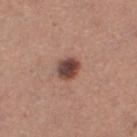| field | value |
|---|---|
| biopsy status | imaged on a skin check; not biopsied |
| TBP lesion metrics | an outline eccentricity of about 0.45 (0 = round, 1 = elongated) and two-axis asymmetry of about 0.2 |
| lighting | white-light illumination |
| subject | female, aged 28–32 |
| body site | the right thigh |
| image | 15 mm crop, total-body photography |